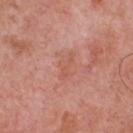No biopsy was performed on this lesion — it was imaged during a full skin examination and was not determined to be concerning. Longest diameter approximately 3.5 mm. A 15 mm close-up tile from a total-body photography series done for melanoma screening. On the chest. Imaged with white-light lighting. The subject is a male about 70 years old.